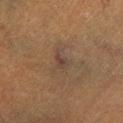Clinical impression: This lesion was catalogued during total-body skin photography and was not selected for biopsy. Acquisition and patient details: The patient is a male aged around 40. The lesion is located on the left lower leg. Captured under cross-polarized illumination. A close-up tile cropped from a whole-body skin photograph, about 15 mm across. The lesion-visualizer software estimated about 5 CIELAB-L* units darker than the surrounding skin and a normalized lesion–skin contrast near 6. The analysis additionally found a border-irregularity index near 3.5/10, internal color variation of about 2.5 on a 0–10 scale, and a peripheral color-asymmetry measure near 0.5. The analysis additionally found an automated nevus-likeness rating near 5 out of 100 and a detector confidence of about 80 out of 100 that the crop contains a lesion. Longest diameter approximately 3 mm.